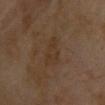Notes:
• biopsy status: imaged on a skin check; not biopsied
• image: 15 mm crop, total-body photography
• image-analysis metrics: a footprint of about 7 mm², an eccentricity of roughly 0.8, and a shape-asymmetry score of about 0.35 (0 = symmetric); a border-irregularity index near 4/10 and a peripheral color-asymmetry measure near 0.5; a classifier nevus-likeness of about 0/100 and a detector confidence of about 100 out of 100 that the crop contains a lesion
• location: the chest
• patient: female, aged 58–62